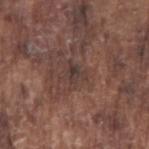Findings:
- workup: total-body-photography surveillance lesion; no biopsy
- image: ~15 mm tile from a whole-body skin photo
- patient: male, about 75 years old
- lesion diameter: ≈3 mm
- automated metrics: a mean CIELAB color near L≈36 a*≈16 b*≈19 and a lesion–skin lightness drop of about 6; border irregularity of about 5 on a 0–10 scale and internal color variation of about 3.5 on a 0–10 scale
- site: the right upper arm